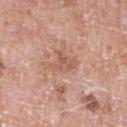Q: Was this lesion biopsied?
A: total-body-photography surveillance lesion; no biopsy
Q: Automated lesion metrics?
A: an average lesion color of about L≈57 a*≈21 b*≈29 (CIELAB) and a lesion–skin lightness drop of about 8; an automated nevus-likeness rating near 0 out of 100
Q: Patient demographics?
A: male, aged 48–52
Q: What kind of image is this?
A: 15 mm crop, total-body photography
Q: Lesion size?
A: ≈4 mm
Q: Lesion location?
A: the left lower leg